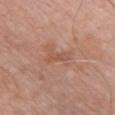{"biopsy_status": "not biopsied; imaged during a skin examination", "lesion_size": {"long_diameter_mm_approx": 3.0}, "lighting": "white-light", "image": {"source": "total-body photography crop", "field_of_view_mm": 15}, "patient": {"sex": "male", "age_approx": 70}, "site": "chest"}